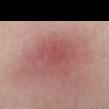Recorded during total-body skin imaging; not selected for excision or biopsy.
Automated tile analysis of the lesion measured a lesion area of about 9.5 mm², an outline eccentricity of about 0.6 (0 = round, 1 = elongated), and a shape-asymmetry score of about 0.25 (0 = symmetric). The software also gave a lesion color around L≈51 a*≈30 b*≈22 in CIELAB, about 6 CIELAB-L* units darker than the surrounding skin, and a normalized border contrast of about 4.5. And it measured a border-irregularity rating of about 3/10, a within-lesion color-variation index near 2.5/10, and a peripheral color-asymmetry measure near 1.
A close-up tile cropped from a whole-body skin photograph, about 15 mm across.
A female subject, aged approximately 40.
The lesion is on the left arm.
About 4 mm across.
The tile uses cross-polarized illumination.